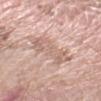Image and clinical context: Imaged with white-light lighting. The lesion is located on the arm. Automated image analysis of the tile measured an average lesion color of about L≈64 a*≈18 b*≈25 (CIELAB), roughly 8 lightness units darker than nearby skin, and a normalized border contrast of about 5. The software also gave border irregularity of about 4.5 on a 0–10 scale, internal color variation of about 3 on a 0–10 scale, and peripheral color asymmetry of about 1. And it measured a classifier nevus-likeness of about 0/100. A 15 mm close-up extracted from a 3D total-body photography capture. Measured at roughly 5.5 mm in maximum diameter. A female patient approximately 75 years of age.